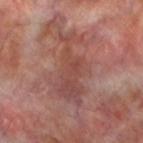The lesion is located on the left lower leg. The lesion-visualizer software estimated a footprint of about 19 mm² and a symmetry-axis asymmetry near 0.5. It also reported border irregularity of about 8.5 on a 0–10 scale, a color-variation rating of about 4/10, and radial color variation of about 1. The patient is a male in their 70s. A roughly 15 mm field-of-view crop from a total-body skin photograph. Longest diameter approximately 8 mm.